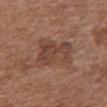workup: imaged on a skin check; not biopsied
patient: female, approximately 75 years of age
image: ~15 mm crop, total-body skin-cancer survey
diameter: ~4.5 mm (longest diameter)
location: the front of the torso
lighting: white-light